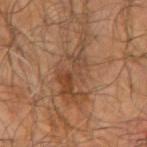No biopsy was performed on this lesion — it was imaged during a full skin examination and was not determined to be concerning.
Located on the left forearm.
A close-up tile cropped from a whole-body skin photograph, about 15 mm across.
The total-body-photography lesion software estimated a mean CIELAB color near L≈42 a*≈19 b*≈30, about 8 CIELAB-L* units darker than the surrounding skin, and a normalized border contrast of about 6.5. The software also gave a nevus-likeness score of about 30/100 and a detector confidence of about 70 out of 100 that the crop contains a lesion.
Measured at roughly 8.5 mm in maximum diameter.
This is a cross-polarized tile.
The subject is a male roughly 65 years of age.